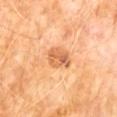| field | value |
|---|---|
| notes | no biopsy performed (imaged during a skin exam) |
| site | the abdomen |
| subject | male, aged 58 to 62 |
| image | 15 mm crop, total-body photography |
| size | ≈3 mm |